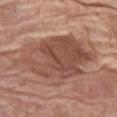biopsy_status: not biopsied; imaged during a skin examination
patient:
  sex: female
  age_approx: 75
site: left thigh
automated_metrics:
  area_mm2_approx: 36.0
  eccentricity: 0.75
  shape_asymmetry: 0.2
  border_irregularity_0_10: 2.5
  color_variation_0_10: 6.0
  peripheral_color_asymmetry: 2.5
lesion_size:
  long_diameter_mm_approx: 9.0
image:
  source: total-body photography crop
  field_of_view_mm: 15
lighting: white-light